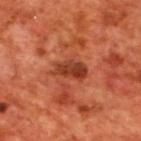Recorded during total-body skin imaging; not selected for excision or biopsy. A male subject, aged 68 to 72. Captured under cross-polarized illumination. The lesion is located on the upper back. A 15 mm crop from a total-body photograph taken for skin-cancer surveillance. Measured at roughly 4 mm in maximum diameter. Automated image analysis of the tile measured an area of roughly 7 mm², a shape eccentricity near 0.85, and a shape-asymmetry score of about 0.25 (0 = symmetric). The analysis additionally found an average lesion color of about L≈39 a*≈31 b*≈35 (CIELAB), a lesion–skin lightness drop of about 11, and a normalized lesion–skin contrast near 9. And it measured a nevus-likeness score of about 5/100.